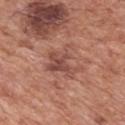biopsy status: no biopsy performed (imaged during a skin exam)
subject: male, roughly 70 years of age
diameter: ≈4.5 mm
location: the mid back
TBP lesion metrics: a lesion color around L≈49 a*≈24 b*≈26 in CIELAB
imaging modality: ~15 mm crop, total-body skin-cancer survey
tile lighting: white-light